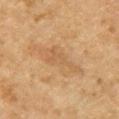Recorded during total-body skin imaging; not selected for excision or biopsy.
A lesion tile, about 15 mm wide, cut from a 3D total-body photograph.
The lesion is on the chest.
A female subject aged approximately 60.
About 5 mm across.